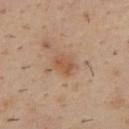Q: Is there a histopathology result?
A: no biopsy performed (imaged during a skin exam)
Q: Who is the patient?
A: male, in their 40s
Q: What is the anatomic site?
A: the upper back
Q: How was this image acquired?
A: 15 mm crop, total-body photography